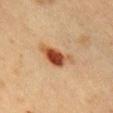Clinical impression:
The lesion was photographed on a routine skin check and not biopsied; there is no pathology result.
Clinical summary:
From the chest. A lesion tile, about 15 mm wide, cut from a 3D total-body photograph. A female patient approximately 40 years of age. Imaged with cross-polarized lighting. The lesion's longest dimension is about 5 mm.